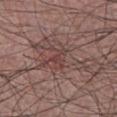This image is a 15 mm lesion crop taken from a total-body photograph. The lesion is on the chest. Approximately 2.5 mm at its widest. This is a white-light tile. A male patient approximately 40 years of age.